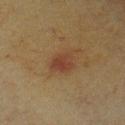Captured during whole-body skin photography for melanoma surveillance; the lesion was not biopsied.
About 3 mm across.
Imaged with cross-polarized lighting.
On the chest.
An algorithmic analysis of the crop reported an area of roughly 6.5 mm², a shape eccentricity near 0.6, and a symmetry-axis asymmetry near 0.2. The software also gave a mean CIELAB color near L≈32 a*≈17 b*≈25 and about 7 CIELAB-L* units darker than the surrounding skin. It also reported a nevus-likeness score of about 20/100.
The subject is a male aged 63–67.
Cropped from a whole-body photographic skin survey; the tile spans about 15 mm.